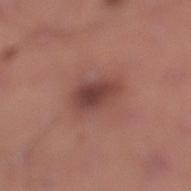workup = total-body-photography surveillance lesion; no biopsy | subject = male, approximately 30 years of age | acquisition = total-body-photography crop, ~15 mm field of view | body site = the left lower leg.